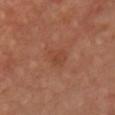Case summary:
• notes · imaged on a skin check; not biopsied
• location · the chest
• tile lighting · cross-polarized illumination
• acquisition · ~15 mm crop, total-body skin-cancer survey
• subject · male, about 65 years old
• automated lesion analysis · a mean CIELAB color near L≈44 a*≈25 b*≈32 and about 5 CIELAB-L* units darker than the surrounding skin; a nevus-likeness score of about 0/100 and lesion-presence confidence of about 100/100
• lesion size · ~3 mm (longest diameter)